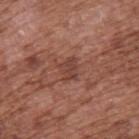follow-up=catalogued during a skin exam; not biopsied
subject=male, aged 73 to 77
anatomic site=the upper back
imaging modality=~15 mm crop, total-body skin-cancer survey
tile lighting=white-light
image-analysis metrics=a nevus-likeness score of about 0/100 and a detector confidence of about 100 out of 100 that the crop contains a lesion
lesion size=≈3 mm